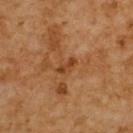The lesion was photographed on a routine skin check and not biopsied; there is no pathology result.
This image is a 15 mm lesion crop taken from a total-body photograph.
A male subject aged around 60.
Approximately 2.5 mm at its widest.
This is a cross-polarized tile.
From the upper back.